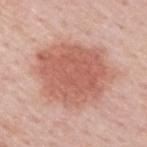Imaged with white-light lighting. On the back. A male patient, in their 60s. A close-up tile cropped from a whole-body skin photograph, about 15 mm across.